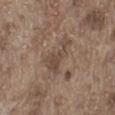<record>
  <patient>
    <sex>male</sex>
    <age_approx>70</age_approx>
  </patient>
  <image>
    <source>total-body photography crop</source>
    <field_of_view_mm>15</field_of_view_mm>
  </image>
  <site>leg</site>
  <lighting>white-light</lighting>
  <lesion_size>
    <long_diameter_mm_approx>4.5</long_diameter_mm_approx>
  </lesion_size>
</record>